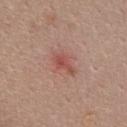The lesion was tiled from a total-body skin photograph and was not biopsied. A female patient, about 50 years old. The lesion is located on the front of the torso. Imaged with white-light lighting. About 3 mm across. This image is a 15 mm lesion crop taken from a total-body photograph.